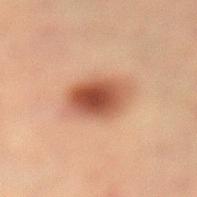biopsy status — imaged on a skin check; not biopsied | image source — total-body-photography crop, ~15 mm field of view | tile lighting — cross-polarized | size — ~5 mm (longest diameter) | location — the left lower leg | subject — female, roughly 55 years of age | TBP lesion metrics — an average lesion color of about L≈42 a*≈21 b*≈28 (CIELAB), roughly 13 lightness units darker than nearby skin, and a normalized lesion–skin contrast near 10.5; an automated nevus-likeness rating near 100 out of 100 and lesion-presence confidence of about 100/100.